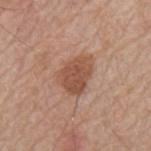<lesion>
<biopsy_status>not biopsied; imaged during a skin examination</biopsy_status>
</lesion>